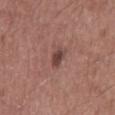Q: Is there a histopathology result?
A: total-body-photography surveillance lesion; no biopsy
Q: What are the patient's age and sex?
A: male, aged 58 to 62
Q: What is the imaging modality?
A: ~15 mm tile from a whole-body skin photo
Q: What is the anatomic site?
A: the back
Q: How was the tile lit?
A: white-light illumination
Q: Lesion size?
A: about 3 mm